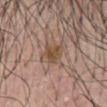- follow-up: catalogued during a skin exam; not biopsied
- illumination: white-light illumination
- image: ~15 mm crop, total-body skin-cancer survey
- lesion diameter: ≈3 mm
- site: the abdomen
- patient: male, aged around 55